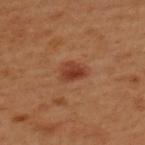Q: Was this lesion biopsied?
A: total-body-photography surveillance lesion; no biopsy
Q: Illumination type?
A: cross-polarized
Q: Patient demographics?
A: male, in their 50s
Q: Lesion location?
A: the upper back
Q: What did automated image analysis measure?
A: an area of roughly 4.5 mm² and two-axis asymmetry of about 0.35; a mean CIELAB color near L≈39 a*≈27 b*≈32 and a normalized border contrast of about 8.5; border irregularity of about 3 on a 0–10 scale, a color-variation rating of about 4/10, and peripheral color asymmetry of about 1.5
Q: What kind of image is this?
A: ~15 mm tile from a whole-body skin photo
Q: How large is the lesion?
A: ~2.5 mm (longest diameter)